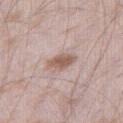image: 15 mm crop, total-body photography
size: ≈3.5 mm
automated lesion analysis: an average lesion color of about L≈57 a*≈18 b*≈25 (CIELAB), roughly 12 lightness units darker than nearby skin, and a normalized border contrast of about 8; a within-lesion color-variation index near 2/10
subject: male, in their mid-40s
illumination: white-light illumination
location: the right thigh